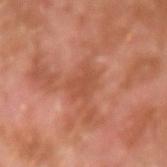Recorded during total-body skin imaging; not selected for excision or biopsy. The recorded lesion diameter is about 3.5 mm. This image is a 15 mm lesion crop taken from a total-body photograph. A male subject roughly 30 years of age. The lesion is located on the left forearm.